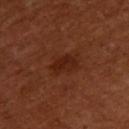notes = no biopsy performed (imaged during a skin exam)
location = the upper back
diameter = about 3.5 mm
subject = female, approximately 55 years of age
automated metrics = a border-irregularity rating of about 3/10, a color-variation rating of about 2.5/10, and a peripheral color-asymmetry measure near 1; a lesion-detection confidence of about 100/100
illumination = cross-polarized illumination
imaging modality = ~15 mm crop, total-body skin-cancer survey